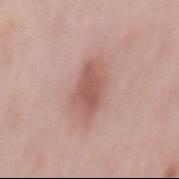Part of a total-body skin-imaging series; this lesion was reviewed on a skin check and was not flagged for biopsy.
Located on the back.
Captured under white-light illumination.
The subject is a female approximately 65 years of age.
Cropped from a whole-body photographic skin survey; the tile spans about 15 mm.
Automated image analysis of the tile measured a lesion area of about 11 mm², an eccentricity of roughly 0.85, and a symmetry-axis asymmetry near 0.2.